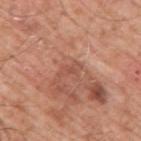biopsy status: catalogued during a skin exam; not biopsied
diameter: about 2.5 mm
body site: the right upper arm
illumination: white-light illumination
imaging modality: total-body-photography crop, ~15 mm field of view
patient: male, roughly 60 years of age
image-analysis metrics: a lesion color around L≈52 a*≈25 b*≈30 in CIELAB and roughly 7 lightness units darker than nearby skin; a border-irregularity rating of about 4.5/10 and a within-lesion color-variation index near 0/10; an automated nevus-likeness rating near 0 out of 100